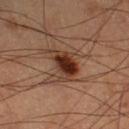Impression: Captured during whole-body skin photography for melanoma surveillance; the lesion was not biopsied. Acquisition and patient details: A lesion tile, about 15 mm wide, cut from a 3D total-body photograph. On the leg. A male patient aged approximately 60.